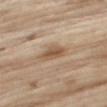No biopsy was performed on this lesion — it was imaged during a full skin examination and was not determined to be concerning. From the right thigh. A male patient aged 68 to 72. A roughly 15 mm field-of-view crop from a total-body skin photograph.